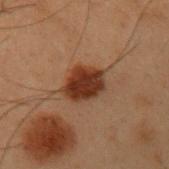  biopsy_status: not biopsied; imaged during a skin examination
  patient:
    sex: male
    age_approx: 55
  automated_metrics:
    cielab_L: 28
    cielab_a: 20
    cielab_b: 26
    vs_skin_darker_L: 13.0
  lesion_size:
    long_diameter_mm_approx: 4.5
  site: left upper arm
  image:
    source: total-body photography crop
    field_of_view_mm: 15